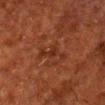<lesion>
<biopsy_status>not biopsied; imaged during a skin examination</biopsy_status>
<automated_metrics>
  <color_variation_0_10>1.0</color_variation_0_10>
  <peripheral_color_asymmetry>0.5</peripheral_color_asymmetry>
</automated_metrics>
<lesion_size>
  <long_diameter_mm_approx>4.0</long_diameter_mm_approx>
</lesion_size>
<patient>
  <sex>male</sex>
  <age_approx>60</age_approx>
</patient>
<lighting>cross-polarized</lighting>
<site>head or neck</site>
<image>
  <source>total-body photography crop</source>
  <field_of_view_mm>15</field_of_view_mm>
</image>
</lesion>